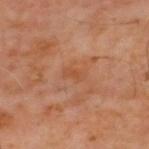Impression:
The lesion was tiled from a total-body skin photograph and was not biopsied.
Background:
The patient is a male aged 58–62. On the upper back. A region of skin cropped from a whole-body photographic capture, roughly 15 mm wide.